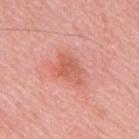Clinical impression: The lesion was photographed on a routine skin check and not biopsied; there is no pathology result. Acquisition and patient details: The recorded lesion diameter is about 3.5 mm. A male patient, aged 48 to 52. From the mid back. A lesion tile, about 15 mm wide, cut from a 3D total-body photograph.